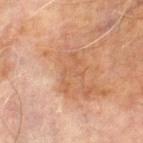Assessment:
No biopsy was performed on this lesion — it was imaged during a full skin examination and was not determined to be concerning.
Acquisition and patient details:
Measured at roughly 5 mm in maximum diameter. A male subject, aged approximately 70. The lesion is located on the left lower leg. A roughly 15 mm field-of-view crop from a total-body skin photograph.